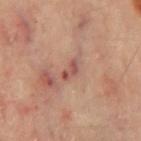illumination: cross-polarized | lesion size: about 2.5 mm | subject: male, aged 63–67 | image: total-body-photography crop, ~15 mm field of view | automated lesion analysis: an outline eccentricity of about 0.9 (0 = round, 1 = elongated) and two-axis asymmetry of about 0.5 | body site: the back.